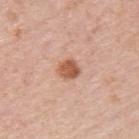Recorded during total-body skin imaging; not selected for excision or biopsy. The lesion is on the upper back. Captured under white-light illumination. A female subject aged 53–57. A roughly 15 mm field-of-view crop from a total-body skin photograph. The lesion's longest dimension is about 2.5 mm.